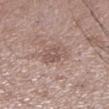The lesion was photographed on a routine skin check and not biopsied; there is no pathology result.
Captured under white-light illumination.
From the left lower leg.
A close-up tile cropped from a whole-body skin photograph, about 15 mm across.
A male subject aged 48–52.
The lesion-visualizer software estimated a footprint of about 5.5 mm², an outline eccentricity of about 0.75 (0 = round, 1 = elongated), and two-axis asymmetry of about 0.3. It also reported a lesion color around L≈54 a*≈17 b*≈23 in CIELAB and a lesion-to-skin contrast of about 5.5 (normalized; higher = more distinct). The software also gave a border-irregularity rating of about 3/10, a within-lesion color-variation index near 3/10, and a peripheral color-asymmetry measure near 1. And it measured an automated nevus-likeness rating near 0 out of 100.